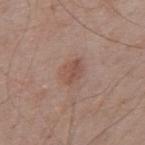Impression:
The lesion was photographed on a routine skin check and not biopsied; there is no pathology result.
Background:
The subject is a male in their 70s. About 3 mm across. A close-up tile cropped from a whole-body skin photograph, about 15 mm across. This is a white-light tile. The total-body-photography lesion software estimated an area of roughly 5.5 mm², a shape eccentricity near 0.7, and two-axis asymmetry of about 0.25. It also reported an average lesion color of about L≈51 a*≈20 b*≈26 (CIELAB) and roughly 7 lightness units darker than nearby skin. It also reported a border-irregularity rating of about 2.5/10 and internal color variation of about 3 on a 0–10 scale. It also reported an automated nevus-likeness rating near 25 out of 100 and a detector confidence of about 100 out of 100 that the crop contains a lesion. The lesion is on the upper back.